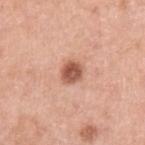Part of a total-body skin-imaging series; this lesion was reviewed on a skin check and was not flagged for biopsy. Captured under white-light illumination. From the left upper arm. A region of skin cropped from a whole-body photographic capture, roughly 15 mm wide. The total-body-photography lesion software estimated an area of roughly 5 mm² and a shape eccentricity near 0.5. And it measured a mean CIELAB color near L≈55 a*≈25 b*≈30, roughly 16 lightness units darker than nearby skin, and a lesion-to-skin contrast of about 10 (normalized; higher = more distinct). The analysis additionally found border irregularity of about 1.5 on a 0–10 scale, a within-lesion color-variation index near 3.5/10, and peripheral color asymmetry of about 1.5. And it measured a nevus-likeness score of about 90/100 and a detector confidence of about 100 out of 100 that the crop contains a lesion. A male subject, approximately 60 years of age. The lesion's longest dimension is about 2.5 mm.